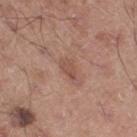The lesion-visualizer software estimated a mean CIELAB color near L≈51 a*≈21 b*≈27 and a normalized lesion–skin contrast near 6. The analysis additionally found radial color variation of about 0.
From the right thigh.
The lesion's longest dimension is about 2.5 mm.
The subject is a male aged around 55.
Cropped from a whole-body photographic skin survey; the tile spans about 15 mm.
Captured under white-light illumination.